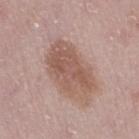Q: Was a biopsy performed?
A: catalogued during a skin exam; not biopsied
Q: Patient demographics?
A: female, about 50 years old
Q: How large is the lesion?
A: ~7 mm (longest diameter)
Q: What is the imaging modality?
A: 15 mm crop, total-body photography
Q: Where on the body is the lesion?
A: the leg